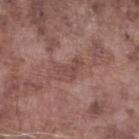Captured during whole-body skin photography for melanoma surveillance; the lesion was not biopsied.